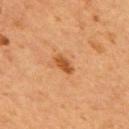<tbp_lesion>
<biopsy_status>not biopsied; imaged during a skin examination</biopsy_status>
<image>
  <source>total-body photography crop</source>
  <field_of_view_mm>15</field_of_view_mm>
</image>
<site>back</site>
<patient>
  <sex>male</sex>
  <age_approx>55</age_approx>
</patient>
<lighting>cross-polarized</lighting>
</tbp_lesion>